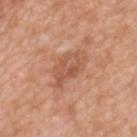Recorded during total-body skin imaging; not selected for excision or biopsy. The total-body-photography lesion software estimated an area of roughly 9.5 mm², a shape eccentricity near 0.9, and two-axis asymmetry of about 0.25. It also reported a mean CIELAB color near L≈55 a*≈24 b*≈32 and roughly 9 lightness units darker than nearby skin. The analysis additionally found a peripheral color-asymmetry measure near 1.5. The analysis additionally found a nevus-likeness score of about 0/100. The lesion's longest dimension is about 5 mm. The patient is a male aged 48–52. Cropped from a whole-body photographic skin survey; the tile spans about 15 mm. From the upper back.